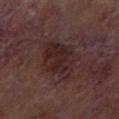Findings:
* biopsy status · total-body-photography surveillance lesion; no biopsy
* site · the right lower leg
* acquisition · total-body-photography crop, ~15 mm field of view
* subject · male, aged around 70
* size · ≈6.5 mm
* tile lighting · cross-polarized illumination